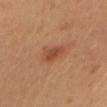Assessment: Part of a total-body skin-imaging series; this lesion was reviewed on a skin check and was not flagged for biopsy. Acquisition and patient details: A female patient in their mid- to late 30s. This is a cross-polarized tile. A 15 mm close-up extracted from a 3D total-body photography capture. The lesion is on the head or neck. Approximately 3.5 mm at its widest.